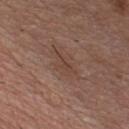<lesion>
<biopsy_status>not biopsied; imaged during a skin examination</biopsy_status>
<image>
  <source>total-body photography crop</source>
  <field_of_view_mm>15</field_of_view_mm>
</image>
<patient>
  <sex>male</sex>
  <age_approx>55</age_approx>
</patient>
<site>chest</site>
</lesion>